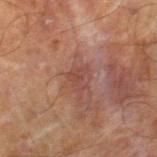Impression: This lesion was catalogued during total-body skin photography and was not selected for biopsy. Clinical summary: Cropped from a total-body skin-imaging series; the visible field is about 15 mm. The total-body-photography lesion software estimated an area of roughly 4 mm² and an eccentricity of roughly 0.65. The analysis additionally found an average lesion color of about L≈37 a*≈20 b*≈22 (CIELAB), roughly 6 lightness units darker than nearby skin, and a normalized lesion–skin contrast near 5. It also reported a nevus-likeness score of about 0/100. A male patient aged approximately 70. The recorded lesion diameter is about 3 mm. The tile uses cross-polarized illumination. The lesion is located on the right thigh.